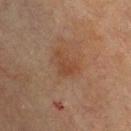Case summary:
– subject: male, in their 70s
– image-analysis metrics: an eccentricity of roughly 0.8 and two-axis asymmetry of about 0.55
– tile lighting: cross-polarized illumination
– site: the chest
– image: total-body-photography crop, ~15 mm field of view
– diameter: ≈3 mm